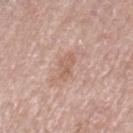| feature | finding |
|---|---|
| subject | female, aged 73 to 77 |
| acquisition | ~15 mm crop, total-body skin-cancer survey |
| size | about 3.5 mm |
| site | the left thigh |
| automated lesion analysis | an average lesion color of about L≈60 a*≈20 b*≈28 (CIELAB), about 8 CIELAB-L* units darker than the surrounding skin, and a lesion-to-skin contrast of about 5.5 (normalized; higher = more distinct) |
| tile lighting | white-light |